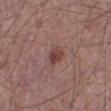follow-up = catalogued during a skin exam; not biopsied | patient = male, in their mid- to late 60s | imaging modality = total-body-photography crop, ~15 mm field of view | tile lighting = white-light | lesion diameter = ≈2.5 mm | location = the right lower leg.